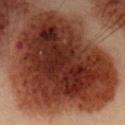Q: Was a biopsy performed?
A: no biopsy performed (imaged during a skin exam)
Q: What is the imaging modality?
A: 15 mm crop, total-body photography
Q: Who is the patient?
A: male, in their mid-50s
Q: How large is the lesion?
A: ≈14.5 mm
Q: Where on the body is the lesion?
A: the abdomen